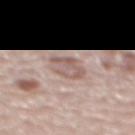• biopsy status · catalogued during a skin exam; not biopsied